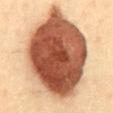This lesion was catalogued during total-body skin photography and was not selected for biopsy.
About 13 mm across.
A close-up tile cropped from a whole-body skin photograph, about 15 mm across.
On the abdomen.
A male subject in their 40s.
The tile uses cross-polarized illumination.